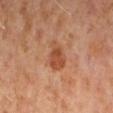biopsy status: catalogued during a skin exam; not biopsied
subject: female, roughly 50 years of age
image-analysis metrics: an area of roughly 7 mm² and a symmetry-axis asymmetry near 0.3; an average lesion color of about L≈48 a*≈25 b*≈34 (CIELAB), a lesion–skin lightness drop of about 9, and a lesion-to-skin contrast of about 7.5 (normalized; higher = more distinct); a lesion-detection confidence of about 100/100
anatomic site: the arm
tile lighting: cross-polarized
acquisition: 15 mm crop, total-body photography